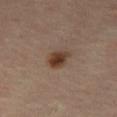follow-up = imaged on a skin check; not biopsied | lighting = cross-polarized illumination | location = the right thigh | subject = male, aged 63–67 | automated lesion analysis = a mean CIELAB color near L≈38 a*≈17 b*≈26, about 12 CIELAB-L* units darker than the surrounding skin, and a normalized lesion–skin contrast near 10.5 | acquisition = total-body-photography crop, ~15 mm field of view.